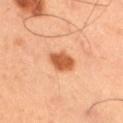Clinical impression:
The lesion was tiled from a total-body skin photograph and was not biopsied.
Context:
A male subject, aged 48 to 52. Located on the right thigh. A close-up tile cropped from a whole-body skin photograph, about 15 mm across. Automated image analysis of the tile measured a normalized lesion–skin contrast near 9.5. The software also gave a nevus-likeness score of about 95/100 and a detector confidence of about 100 out of 100 that the crop contains a lesion.